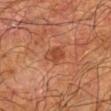Part of a total-body skin-imaging series; this lesion was reviewed on a skin check and was not flagged for biopsy.
Approximately 2.5 mm at its widest.
A male subject approximately 65 years of age.
The lesion is on the arm.
Automated tile analysis of the lesion measured a within-lesion color-variation index near 3/10. And it measured an automated nevus-likeness rating near 60 out of 100 and a lesion-detection confidence of about 100/100.
This is a cross-polarized tile.
A region of skin cropped from a whole-body photographic capture, roughly 15 mm wide.